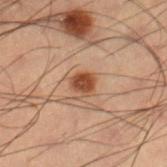Assessment: Captured during whole-body skin photography for melanoma surveillance; the lesion was not biopsied. Image and clinical context: The subject is a male about 55 years old. The total-body-photography lesion software estimated border irregularity of about 1.5 on a 0–10 scale and a color-variation rating of about 3.5/10. It also reported a classifier nevus-likeness of about 95/100 and a lesion-detection confidence of about 100/100. From the right lower leg. The lesion's longest dimension is about 2.5 mm. Cropped from a whole-body photographic skin survey; the tile spans about 15 mm. This is a cross-polarized tile.